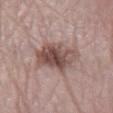The lesion was tiled from a total-body skin photograph and was not biopsied.
Automated tile analysis of the lesion measured a footprint of about 16 mm² and a symmetry-axis asymmetry near 0.2. The software also gave border irregularity of about 3 on a 0–10 scale and a color-variation rating of about 7/10. The analysis additionally found a classifier nevus-likeness of about 65/100 and a lesion-detection confidence of about 100/100.
About 4.5 mm across.
The lesion is located on the left lower leg.
A lesion tile, about 15 mm wide, cut from a 3D total-body photograph.
A male subject approximately 60 years of age.
Captured under white-light illumination.